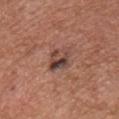notes = no biopsy performed (imaged during a skin exam)
site = the chest
image source = 15 mm crop, total-body photography
patient = male, aged 73–77
diameter = about 3.5 mm